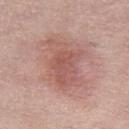<tbp_lesion>
  <biopsy_status>not biopsied; imaged during a skin examination</biopsy_status>
  <lighting>white-light</lighting>
  <site>leg</site>
  <image>
    <source>total-body photography crop</source>
    <field_of_view_mm>15</field_of_view_mm>
  </image>
  <patient>
    <sex>female</sex>
    <age_approx>55</age_approx>
  </patient>
</tbp_lesion>